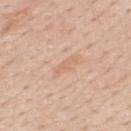| field | value |
|---|---|
| notes | catalogued during a skin exam; not biopsied |
| diameter | ~3 mm (longest diameter) |
| TBP lesion metrics | a footprint of about 2.5 mm², a shape eccentricity near 0.95, and a shape-asymmetry score of about 0.35 (0 = symmetric); border irregularity of about 5 on a 0–10 scale, internal color variation of about 0 on a 0–10 scale, and radial color variation of about 0 |
| image | ~15 mm crop, total-body skin-cancer survey |
| site | the mid back |
| illumination | white-light illumination |
| subject | male, roughly 60 years of age |